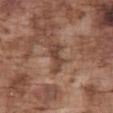automated metrics: an area of roughly 5 mm², an outline eccentricity of about 0.9 (0 = round, 1 = elongated), and two-axis asymmetry of about 0.45; an average lesion color of about L≈43 a*≈20 b*≈27 (CIELAB), about 10 CIELAB-L* units darker than the surrounding skin, and a normalized border contrast of about 8; border irregularity of about 5.5 on a 0–10 scale, internal color variation of about 1 on a 0–10 scale, and peripheral color asymmetry of about 0.5; a nevus-likeness score of about 0/100 and a detector confidence of about 95 out of 100 that the crop contains a lesion | subject: male, aged around 75 | image source: ~15 mm crop, total-body skin-cancer survey | lesion diameter: ≈4 mm | lighting: white-light | site: the abdomen.